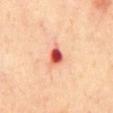follow-up = no biopsy performed (imaged during a skin exam) | tile lighting = cross-polarized | site = the front of the torso | diameter = ≈3 mm | subject = male, approximately 70 years of age | image source = total-body-photography crop, ~15 mm field of view.